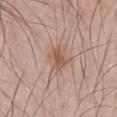{
  "biopsy_status": "not biopsied; imaged during a skin examination",
  "image": {
    "source": "total-body photography crop",
    "field_of_view_mm": 15
  },
  "lighting": "white-light",
  "lesion_size": {
    "long_diameter_mm_approx": 3.0
  },
  "patient": {
    "sex": "male",
    "age_approx": 25
  },
  "site": "chest"
}